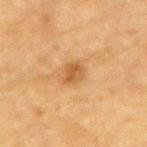Findings:
* follow-up · imaged on a skin check; not biopsied
* tile lighting · cross-polarized illumination
* patient · male, in their mid- to late 80s or older
* imaging modality · 15 mm crop, total-body photography
* anatomic site · the mid back
* image-analysis metrics · an outline eccentricity of about 0.65 (0 = round, 1 = elongated) and two-axis asymmetry of about 0.25; internal color variation of about 3 on a 0–10 scale and peripheral color asymmetry of about 1